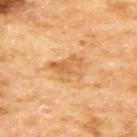| field | value |
|---|---|
| follow-up | no biopsy performed (imaged during a skin exam) |
| lesion diameter | ≈4.5 mm |
| image source | 15 mm crop, total-body photography |
| subject | female, aged approximately 60 |
| lighting | cross-polarized illumination |
| site | the upper back |